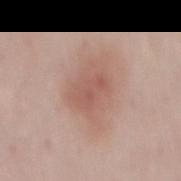Recorded during total-body skin imaging; not selected for excision or biopsy.
The lesion is located on the mid back.
Cropped from a total-body skin-imaging series; the visible field is about 15 mm.
A female patient, aged 48–52.